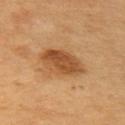Imaged during a routine full-body skin examination; the lesion was not biopsied and no histopathology is available.
A 15 mm crop from a total-body photograph taken for skin-cancer surveillance.
Longest diameter approximately 5 mm.
The lesion is located on the arm.
Captured under cross-polarized illumination.
The patient is a male about 60 years old.